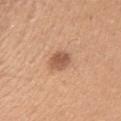{"biopsy_status": "not biopsied; imaged during a skin examination", "site": "right upper arm", "image": {"source": "total-body photography crop", "field_of_view_mm": 15}, "patient": {"sex": "female", "age_approx": 20}}